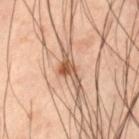Q: Is there a histopathology result?
A: imaged on a skin check; not biopsied
Q: What is the imaging modality?
A: 15 mm crop, total-body photography
Q: Lesion location?
A: the left thigh
Q: What are the patient's age and sex?
A: male, in their 60s
Q: Lesion size?
A: ≈5 mm
Q: Illumination type?
A: cross-polarized illumination
Q: What did automated image analysis measure?
A: a lesion color around L≈56 a*≈20 b*≈31 in CIELAB, a lesion–skin lightness drop of about 13, and a normalized lesion–skin contrast near 8.5; a detector confidence of about 100 out of 100 that the crop contains a lesion